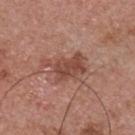{
  "biopsy_status": "not biopsied; imaged during a skin examination",
  "lighting": "white-light",
  "image": {
    "source": "total-body photography crop",
    "field_of_view_mm": 15
  },
  "site": "chest",
  "patient": {
    "sex": "male",
    "age_approx": 55
  }
}